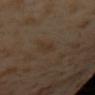The lesion was photographed on a routine skin check and not biopsied; there is no pathology result.
A female subject approximately 55 years of age.
Measured at roughly 3 mm in maximum diameter.
From the mid back.
A lesion tile, about 15 mm wide, cut from a 3D total-body photograph.